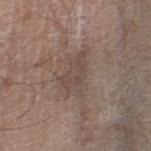Captured during whole-body skin photography for melanoma surveillance; the lesion was not biopsied. Located on the right lower leg. Automated tile analysis of the lesion measured a border-irregularity index near 5/10 and internal color variation of about 2.5 on a 0–10 scale. Longest diameter approximately 5 mm. The patient is a male aged 58 to 62. A roughly 15 mm field-of-view crop from a total-body skin photograph.